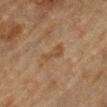Case summary:
• workup — imaged on a skin check; not biopsied
• body site — the left lower leg
• subject — male, aged 83 or older
• lesion size — about 3.5 mm
• lighting — cross-polarized illumination
• image-analysis metrics — an area of roughly 4 mm², an outline eccentricity of about 0.9 (0 = round, 1 = elongated), and a shape-asymmetry score of about 0.45 (0 = symmetric)
• image — 15 mm crop, total-body photography